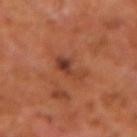Impression: No biopsy was performed on this lesion — it was imaged during a full skin examination and was not determined to be concerning. Clinical summary: About 3 mm across. Cropped from a total-body skin-imaging series; the visible field is about 15 mm. A male patient aged 58–62. Captured under cross-polarized illumination. Automated image analysis of the tile measured a footprint of about 5.5 mm², an eccentricity of roughly 0.7, and a symmetry-axis asymmetry near 0.5. The software also gave a lesion color around L≈37 a*≈25 b*≈30 in CIELAB, roughly 8 lightness units darker than nearby skin, and a lesion-to-skin contrast of about 7.5 (normalized; higher = more distinct). It also reported a border-irregularity index near 5.5/10 and a within-lesion color-variation index near 5.5/10. And it measured an automated nevus-likeness rating near 0 out of 100. The lesion is located on the left forearm.